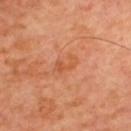<lesion>
<biopsy_status>not biopsied; imaged during a skin examination</biopsy_status>
<image>
  <source>total-body photography crop</source>
  <field_of_view_mm>15</field_of_view_mm>
</image>
<site>upper back</site>
<lesion_size>
  <long_diameter_mm_approx>3.0</long_diameter_mm_approx>
</lesion_size>
<lighting>cross-polarized</lighting>
<patient>
  <sex>male</sex>
  <age_approx>60</age_approx>
</patient>
</lesion>